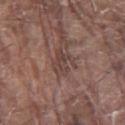This lesion was catalogued during total-body skin photography and was not selected for biopsy.
A roughly 15 mm field-of-view crop from a total-body skin photograph.
The lesion is located on the right thigh.
A male patient aged 78–82.
About 3 mm across.